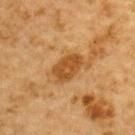Case summary:
- follow-up — catalogued during a skin exam; not biopsied
- diameter — ~3.5 mm (longest diameter)
- automated lesion analysis — a lesion area of about 7 mm², an outline eccentricity of about 0.75 (0 = round, 1 = elongated), and two-axis asymmetry of about 0.2
- subject — male, in their mid- to late 80s or older
- site — the upper back
- imaging modality — ~15 mm crop, total-body skin-cancer survey
- lighting — cross-polarized illumination